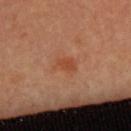This lesion was catalogued during total-body skin photography and was not selected for biopsy.
Captured under cross-polarized illumination.
A roughly 15 mm field-of-view crop from a total-body skin photograph.
About 3 mm across.
A female subject, in their 50s.